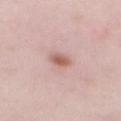Clinical summary: The lesion's longest dimension is about 3 mm. This image is a 15 mm lesion crop taken from a total-body photograph. An algorithmic analysis of the crop reported an eccentricity of roughly 0.85 and a shape-asymmetry score of about 0.15 (0 = symmetric). And it measured a lesion color around L≈60 a*≈22 b*≈25 in CIELAB, roughly 12 lightness units darker than nearby skin, and a lesion-to-skin contrast of about 8 (normalized; higher = more distinct). It also reported border irregularity of about 2 on a 0–10 scale, a color-variation rating of about 3.5/10, and peripheral color asymmetry of about 1. The analysis additionally found an automated nevus-likeness rating near 95 out of 100 and a detector confidence of about 100 out of 100 that the crop contains a lesion. The lesion is located on the mid back. A female subject, in their 40s.